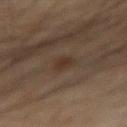• follow-up: total-body-photography surveillance lesion; no biopsy
• automated lesion analysis: a lesion area of about 4 mm² and a symmetry-axis asymmetry near 0.3; a lesion color around L≈29 a*≈13 b*≈22 in CIELAB, roughly 5 lightness units darker than nearby skin, and a lesion-to-skin contrast of about 6 (normalized; higher = more distinct); internal color variation of about 1.5 on a 0–10 scale and peripheral color asymmetry of about 0.5; an automated nevus-likeness rating near 60 out of 100 and lesion-presence confidence of about 100/100
• subject: male, in their 50s
• lesion diameter: ≈2.5 mm
• location: the right upper arm
• imaging modality: 15 mm crop, total-body photography
• illumination: cross-polarized illumination